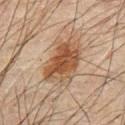This lesion was catalogued during total-body skin photography and was not selected for biopsy. From the abdomen. Approximately 5 mm at its widest. Captured under cross-polarized illumination. The lesion-visualizer software estimated a shape eccentricity near 0.75 and a shape-asymmetry score of about 0.3 (0 = symmetric). The analysis additionally found border irregularity of about 3 on a 0–10 scale, a within-lesion color-variation index near 4/10, and peripheral color asymmetry of about 1.5. The software also gave a nevus-likeness score of about 95/100. A male patient aged approximately 70. A region of skin cropped from a whole-body photographic capture, roughly 15 mm wide.